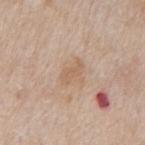image-analysis metrics: an area of roughly 3.5 mm², an eccentricity of roughly 0.85, and a shape-asymmetry score of about 0.45 (0 = symmetric); a nevus-likeness score of about 0/100 and lesion-presence confidence of about 100/100 | acquisition: ~15 mm tile from a whole-body skin photo | anatomic site: the mid back | tile lighting: white-light illumination | diameter: ≈2.5 mm | patient: male, aged 63 to 67.